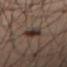notes: imaged on a skin check; not biopsied
subject: male, aged approximately 45
lighting: cross-polarized
acquisition: 15 mm crop, total-body photography
site: the left thigh